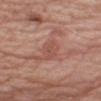Impression:
The lesion was tiled from a total-body skin photograph and was not biopsied.
Context:
The lesion is on the right upper arm. About 3.5 mm across. This image is a 15 mm lesion crop taken from a total-body photograph. An algorithmic analysis of the crop reported a lesion area of about 5.5 mm², an eccentricity of roughly 0.8, and a symmetry-axis asymmetry near 0.25. The software also gave a classifier nevus-likeness of about 5/100 and a detector confidence of about 95 out of 100 that the crop contains a lesion. The patient is a male roughly 75 years of age.